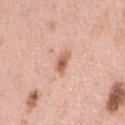Impression:
Recorded during total-body skin imaging; not selected for excision or biopsy.
Acquisition and patient details:
The lesion is located on the right upper arm. A roughly 15 mm field-of-view crop from a total-body skin photograph. A female subject aged 48 to 52.